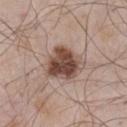notes: total-body-photography surveillance lesion; no biopsy
imaging modality: ~15 mm tile from a whole-body skin photo
site: the chest
lesion diameter: ~5 mm (longest diameter)
image-analysis metrics: an area of roughly 14 mm², an outline eccentricity of about 0.5 (0 = round, 1 = elongated), and a shape-asymmetry score of about 0.25 (0 = symmetric); a lesion color around L≈47 a*≈18 b*≈24 in CIELAB, a lesion–skin lightness drop of about 16, and a normalized lesion–skin contrast near 11.5; a border-irregularity index near 2.5/10, internal color variation of about 6.5 on a 0–10 scale, and peripheral color asymmetry of about 2.5; a classifier nevus-likeness of about 80/100 and lesion-presence confidence of about 100/100
patient: male, aged approximately 55